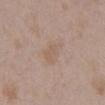Clinical impression: Part of a total-body skin-imaging series; this lesion was reviewed on a skin check and was not flagged for biopsy. Clinical summary: A male subject aged 48 to 52. The lesion is located on the chest. A region of skin cropped from a whole-body photographic capture, roughly 15 mm wide.